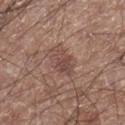Recorded during total-body skin imaging; not selected for excision or biopsy.
A male patient, about 60 years old.
Cropped from a whole-body photographic skin survey; the tile spans about 15 mm.
The lesion is on the right lower leg.